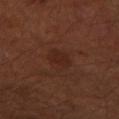Case summary:
- follow-up: total-body-photography surveillance lesion; no biopsy
- site: the left forearm
- lighting: cross-polarized illumination
- image-analysis metrics: an area of roughly 6.5 mm² and a shape eccentricity near 0.65; a border-irregularity rating of about 1.5/10, a within-lesion color-variation index near 2/10, and a peripheral color-asymmetry measure near 0.5
- subject: male, aged around 60
- acquisition: ~15 mm tile from a whole-body skin photo